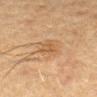The lesion was tiled from a total-body skin photograph and was not biopsied. A close-up tile cropped from a whole-body skin photograph, about 15 mm across. A female patient, aged 48 to 52. The lesion-visualizer software estimated a footprint of about 4.5 mm², a shape eccentricity near 0.65, and a shape-asymmetry score of about 0.4 (0 = symmetric). The analysis additionally found a border-irregularity rating of about 4/10 and internal color variation of about 2 on a 0–10 scale. The software also gave an automated nevus-likeness rating near 25 out of 100 and lesion-presence confidence of about 100/100. Captured under cross-polarized illumination. The lesion's longest dimension is about 3 mm. On the arm.